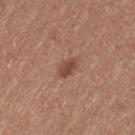follow-up: total-body-photography surveillance lesion; no biopsy | tile lighting: white-light | lesion diameter: ~2.5 mm (longest diameter) | TBP lesion metrics: a footprint of about 3.5 mm², an outline eccentricity of about 0.75 (0 = round, 1 = elongated), and a symmetry-axis asymmetry near 0.15; an average lesion color of about L≈46 a*≈22 b*≈27 (CIELAB), roughly 10 lightness units darker than nearby skin, and a normalized lesion–skin contrast near 8 | image: total-body-photography crop, ~15 mm field of view | anatomic site: the left thigh | subject: female, in their mid-30s.